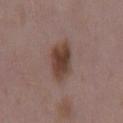Recorded during total-body skin imaging; not selected for excision or biopsy. The patient is a female approximately 35 years of age. About 4.5 mm across. The lesion is on the mid back. A close-up tile cropped from a whole-body skin photograph, about 15 mm across.